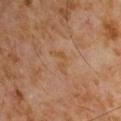Part of a total-body skin-imaging series; this lesion was reviewed on a skin check and was not flagged for biopsy.
Longest diameter approximately 3 mm.
Located on the chest.
A male subject, aged approximately 60.
A 15 mm crop from a total-body photograph taken for skin-cancer surveillance.
This is a cross-polarized tile.